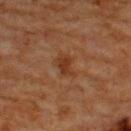Captured during whole-body skin photography for melanoma surveillance; the lesion was not biopsied. The lesion-visualizer software estimated a footprint of about 4.5 mm² and a shape-asymmetry score of about 0.35 (0 = symmetric). It also reported a classifier nevus-likeness of about 0/100 and lesion-presence confidence of about 100/100. From the upper back. This image is a 15 mm lesion crop taken from a total-body photograph. Captured under cross-polarized illumination. The lesion's longest dimension is about 2.5 mm. A male patient, about 65 years old.